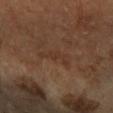| field | value |
|---|---|
| follow-up | imaged on a skin check; not biopsied |
| lesion diameter | ≈4 mm |
| location | the right forearm |
| patient | female, in their 70s |
| illumination | cross-polarized illumination |
| image | total-body-photography crop, ~15 mm field of view |
| TBP lesion metrics | an average lesion color of about L≈35 a*≈18 b*≈28 (CIELAB), roughly 4 lightness units darker than nearby skin, and a normalized border contrast of about 4.5; a border-irregularity rating of about 7.5/10, a color-variation rating of about 1/10, and a peripheral color-asymmetry measure near 0.5; a classifier nevus-likeness of about 0/100 and lesion-presence confidence of about 100/100 |